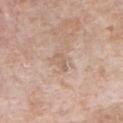notes: catalogued during a skin exam; not biopsied
tile lighting: white-light
subject: male, approximately 60 years of age
lesion size: ~2.5 mm (longest diameter)
automated lesion analysis: a footprint of about 2 mm², an outline eccentricity of about 0.95 (0 = round, 1 = elongated), and a shape-asymmetry score of about 0.35 (0 = symmetric); radial color variation of about 0; a nevus-likeness score of about 0/100
image: ~15 mm crop, total-body skin-cancer survey
body site: the chest